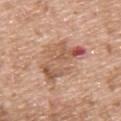Impression:
This lesion was catalogued during total-body skin photography and was not selected for biopsy.
Background:
A lesion tile, about 15 mm wide, cut from a 3D total-body photograph. A male patient roughly 50 years of age. Located on the upper back.